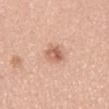Assessment:
Recorded during total-body skin imaging; not selected for excision or biopsy.
Context:
A region of skin cropped from a whole-body photographic capture, roughly 15 mm wide. The lesion is located on the mid back. A male patient about 50 years old.